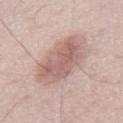* follow-up · catalogued during a skin exam; not biopsied
* size · about 6.5 mm
* subject · male, in their 70s
* site · the mid back
* image source · ~15 mm tile from a whole-body skin photo